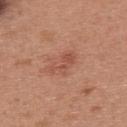Recorded during total-body skin imaging; not selected for excision or biopsy. The lesion-visualizer software estimated an average lesion color of about L≈52 a*≈26 b*≈30 (CIELAB) and a lesion-to-skin contrast of about 5.5 (normalized; higher = more distinct). It also reported border irregularity of about 5 on a 0–10 scale. A region of skin cropped from a whole-body photographic capture, roughly 15 mm wide. The patient is a female about 40 years old. Located on the upper back. Captured under white-light illumination. About 3.5 mm across.